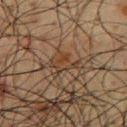follow-up = imaged on a skin check; not biopsied | automated lesion analysis = an eccentricity of roughly 0.8 and two-axis asymmetry of about 0.35; a within-lesion color-variation index near 3.5/10 and radial color variation of about 1; an automated nevus-likeness rating near 0 out of 100 and a lesion-detection confidence of about 55/100 | location = the chest | size = about 3.5 mm | image = total-body-photography crop, ~15 mm field of view | subject = male, aged approximately 60.